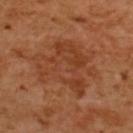Clinical summary:
A female patient roughly 55 years of age. A lesion tile, about 15 mm wide, cut from a 3D total-body photograph. About 8.5 mm across. The lesion is on the upper back. This is a cross-polarized tile. An algorithmic analysis of the crop reported an area of roughly 31 mm², a shape eccentricity near 0.65, and two-axis asymmetry of about 0.35. The software also gave a mean CIELAB color near L≈42 a*≈26 b*≈35 and a lesion-to-skin contrast of about 5.5 (normalized; higher = more distinct). It also reported a border-irregularity index near 6/10 and a within-lesion color-variation index near 5/10. The software also gave a classifier nevus-likeness of about 0/100.